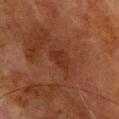biopsy_status: not biopsied; imaged during a skin examination
image:
  source: total-body photography crop
  field_of_view_mm: 15
patient:
  sex: male
  age_approx: 80
automated_metrics:
  border_irregularity_0_10: 3.5
  color_variation_0_10: 0.5
  peripheral_color_asymmetry: 0.0
  nevus_likeness_0_100: 5
  lesion_detection_confidence_0_100: 100
site: chest